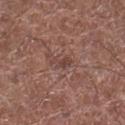A female patient in their 50s. A close-up tile cropped from a whole-body skin photograph, about 15 mm across. An algorithmic analysis of the crop reported an area of roughly 3 mm², a shape eccentricity near 0.9, and a shape-asymmetry score of about 0.45 (0 = symmetric). The software also gave a border-irregularity index near 4.5/10, internal color variation of about 0 on a 0–10 scale, and peripheral color asymmetry of about 0. The software also gave a classifier nevus-likeness of about 0/100 and a lesion-detection confidence of about 90/100. Approximately 2.5 mm at its widest. The lesion is on the right lower leg. The tile uses white-light illumination.